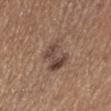Imaged during a routine full-body skin examination; the lesion was not biopsied and no histopathology is available.
The lesion is on the front of the torso.
Measured at roughly 5 mm in maximum diameter.
Automated image analysis of the tile measured a lesion area of about 12 mm² and two-axis asymmetry of about 0.35. It also reported a mean CIELAB color near L≈46 a*≈16 b*≈24, about 9 CIELAB-L* units darker than the surrounding skin, and a normalized border contrast of about 7. It also reported border irregularity of about 4 on a 0–10 scale, a color-variation rating of about 6.5/10, and peripheral color asymmetry of about 2.5.
The subject is a male aged around 65.
A region of skin cropped from a whole-body photographic capture, roughly 15 mm wide.